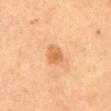<record>
<biopsy_status>not biopsied; imaged during a skin examination</biopsy_status>
<lesion_size>
  <long_diameter_mm_approx>2.5</long_diameter_mm_approx>
</lesion_size>
<image>
  <source>total-body photography crop</source>
  <field_of_view_mm>15</field_of_view_mm>
</image>
<automated_metrics>
  <peripheral_color_asymmetry>1.0</peripheral_color_asymmetry>
  <nevus_likeness_0_100>60</nevus_likeness_0_100>
  <lesion_detection_confidence_0_100>100</lesion_detection_confidence_0_100>
</automated_metrics>
<site>abdomen</site>
<lighting>cross-polarized</lighting>
<patient>
  <sex>female</sex>
  <age_approx>60</age_approx>
</patient>
</record>